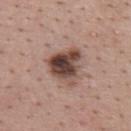{"biopsy_status": "not biopsied; imaged during a skin examination", "patient": {"sex": "male", "age_approx": 25}, "image": {"source": "total-body photography crop", "field_of_view_mm": 15}, "site": "upper back", "lighting": "white-light", "lesion_size": {"long_diameter_mm_approx": 4.5}, "automated_metrics": {"area_mm2_approx": 13.0, "eccentricity": 0.4, "shape_asymmetry": 0.35, "cielab_L": 45, "cielab_a": 18, "cielab_b": 23, "vs_skin_darker_L": 17.0, "vs_skin_contrast_norm": 12.0, "border_irregularity_0_10": 3.5, "color_variation_0_10": 9.5, "peripheral_color_asymmetry": 3.5, "nevus_likeness_0_100": 75, "lesion_detection_confidence_0_100": 100}}